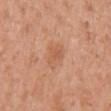Impression:
Part of a total-body skin-imaging series; this lesion was reviewed on a skin check and was not flagged for biopsy.
Image and clinical context:
The lesion is located on the right upper arm. This is a white-light tile. Longest diameter approximately 3.5 mm. A roughly 15 mm field-of-view crop from a total-body skin photograph. A female subject roughly 50 years of age.